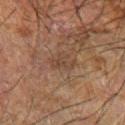Q: How was this image acquired?
A: total-body-photography crop, ~15 mm field of view
Q: What did automated image analysis measure?
A: an area of roughly 4.5 mm² and two-axis asymmetry of about 0.4; an automated nevus-likeness rating near 0 out of 100 and a lesion-detection confidence of about 95/100
Q: How was the tile lit?
A: cross-polarized
Q: What are the patient's age and sex?
A: male, aged around 70
Q: What is the lesion's diameter?
A: ≈3 mm
Q: Where on the body is the lesion?
A: the left forearm